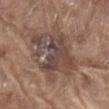Captured during whole-body skin photography for melanoma surveillance; the lesion was not biopsied. A male patient in their 80s. The tile uses white-light illumination. The lesion's longest dimension is about 7 mm. From the abdomen. The total-body-photography lesion software estimated a lesion area of about 26 mm² and a shape-asymmetry score of about 0.4 (0 = symmetric). It also reported an average lesion color of about L≈44 a*≈16 b*≈22 (CIELAB), roughly 9 lightness units darker than nearby skin, and a lesion-to-skin contrast of about 7.5 (normalized; higher = more distinct). The analysis additionally found a within-lesion color-variation index near 7/10 and radial color variation of about 2. The software also gave a classifier nevus-likeness of about 30/100. Cropped from a total-body skin-imaging series; the visible field is about 15 mm.